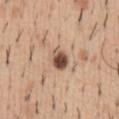Automated tile analysis of the lesion measured a lesion color around L≈51 a*≈19 b*≈28 in CIELAB, roughly 17 lightness units darker than nearby skin, and a lesion-to-skin contrast of about 11.5 (normalized; higher = more distinct). And it measured internal color variation of about 9.5 on a 0–10 scale. The analysis additionally found an automated nevus-likeness rating near 95 out of 100 and a lesion-detection confidence of about 100/100.
A region of skin cropped from a whole-body photographic capture, roughly 15 mm wide.
Imaged with white-light lighting.
A male patient, aged 38–42.
Located on the abdomen.